A close-up tile cropped from a whole-body skin photograph, about 15 mm across · the subject is a female aged approximately 15 · this is a white-light tile · longest diameter approximately 4.5 mm · from the upper back — 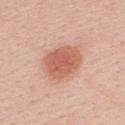Histopathologically confirmed as a dysplastic (Clark) nevus.